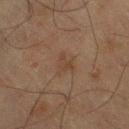Notes:
- biopsy status: no biopsy performed (imaged during a skin exam)
- acquisition: ~15 mm tile from a whole-body skin photo
- tile lighting: cross-polarized illumination
- patient: male, aged approximately 65
- body site: the left thigh
- diameter: ≈3 mm
- TBP lesion metrics: an outline eccentricity of about 0.5 (0 = round, 1 = elongated) and a symmetry-axis asymmetry near 0.35; a border-irregularity rating of about 4/10, a within-lesion color-variation index near 2/10, and radial color variation of about 0.5; a classifier nevus-likeness of about 5/100 and a lesion-detection confidence of about 100/100